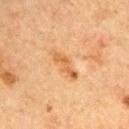{
  "biopsy_status": "not biopsied; imaged during a skin examination",
  "image": {
    "source": "total-body photography crop",
    "field_of_view_mm": 15
  },
  "lighting": "cross-polarized",
  "patient": {
    "sex": "male",
    "age_approx": 65
  },
  "site": "left upper arm",
  "automated_metrics": {
    "area_mm2_approx": 5.5,
    "eccentricity": 0.95,
    "shape_asymmetry": 0.25,
    "border_irregularity_0_10": 4.0,
    "peripheral_color_asymmetry": 0.5,
    "nevus_likeness_0_100": 5
  }
}